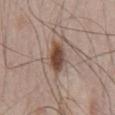Findings:
• biopsy status · imaged on a skin check; not biopsied
• diameter · about 3.5 mm
• body site · the chest
• tile lighting · white-light illumination
• subject · male, in their 50s
• image · ~15 mm crop, total-body skin-cancer survey